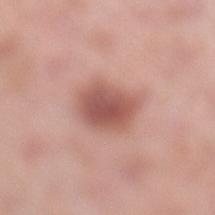Q: What is the imaging modality?
A: total-body-photography crop, ~15 mm field of view
Q: Illumination type?
A: white-light illumination
Q: Lesion size?
A: ~4 mm (longest diameter)
Q: Who is the patient?
A: female, about 50 years old
Q: Lesion location?
A: the left lower leg